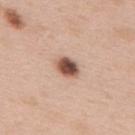<case>
<biopsy_status>not biopsied; imaged during a skin examination</biopsy_status>
<lesion_size>
  <long_diameter_mm_approx>3.5</long_diameter_mm_approx>
</lesion_size>
<image>
  <source>total-body photography crop</source>
  <field_of_view_mm>15</field_of_view_mm>
</image>
<automated_metrics>
  <cielab_L>53</cielab_L>
  <cielab_a>20</cielab_a>
  <cielab_b>27</cielab_b>
  <vs_skin_darker_L>19.0</vs_skin_darker_L>
  <vs_skin_contrast_norm>12.0</vs_skin_contrast_norm>
  <border_irregularity_0_10>1.5</border_irregularity_0_10>
  <color_variation_0_10>6.5</color_variation_0_10>
  <peripheral_color_asymmetry>1.5</peripheral_color_asymmetry>
  <nevus_likeness_0_100>100</nevus_likeness_0_100>
  <lesion_detection_confidence_0_100>100</lesion_detection_confidence_0_100>
</automated_metrics>
<lighting>white-light</lighting>
<site>upper back</site>
<patient>
  <sex>male</sex>
  <age_approx>60</age_approx>
</patient>
</case>